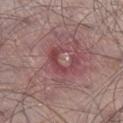| key | value |
|---|---|
| workup | imaged on a skin check; not biopsied |
| patient | male, aged 53–57 |
| lesion size | about 11 mm |
| automated metrics | a border-irregularity rating of about 8/10 and a peripheral color-asymmetry measure near 2; a classifier nevus-likeness of about 0/100 and lesion-presence confidence of about 95/100 |
| image | 15 mm crop, total-body photography |
| anatomic site | the right lower leg |